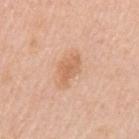Assessment: No biopsy was performed on this lesion — it was imaged during a full skin examination and was not determined to be concerning. Context: This image is a 15 mm lesion crop taken from a total-body photograph. The lesion is on the left upper arm. Imaged with white-light lighting. A female patient, aged approximately 45. Approximately 3.5 mm at its widest.